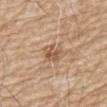Clinical impression:
Captured during whole-body skin photography for melanoma surveillance; the lesion was not biopsied.
Context:
The patient is a male in their 70s. Captured under white-light illumination. The total-body-photography lesion software estimated a lesion area of about 5.5 mm², an outline eccentricity of about 0.75 (0 = round, 1 = elongated), and a shape-asymmetry score of about 0.25 (0 = symmetric). It also reported a lesion color around L≈56 a*≈18 b*≈33 in CIELAB and a lesion-to-skin contrast of about 6.5 (normalized; higher = more distinct). It also reported a border-irregularity rating of about 2.5/10 and peripheral color asymmetry of about 1.5. The analysis additionally found a classifier nevus-likeness of about 0/100 and a lesion-detection confidence of about 100/100. A close-up tile cropped from a whole-body skin photograph, about 15 mm across. Measured at roughly 3 mm in maximum diameter. On the abdomen.